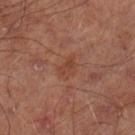Imaged during a routine full-body skin examination; the lesion was not biopsied and no histopathology is available.
An algorithmic analysis of the crop reported an average lesion color of about L≈42 a*≈23 b*≈29 (CIELAB), a lesion–skin lightness drop of about 6, and a lesion-to-skin contrast of about 5.5 (normalized; higher = more distinct). It also reported a border-irregularity rating of about 3.5/10, a color-variation rating of about 2.5/10, and a peripheral color-asymmetry measure near 1.
From the left lower leg.
Captured under cross-polarized illumination.
Measured at roughly 3 mm in maximum diameter.
The patient is a male aged approximately 65.
A lesion tile, about 15 mm wide, cut from a 3D total-body photograph.